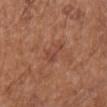{
  "biopsy_status": "not biopsied; imaged during a skin examination",
  "image": {
    "source": "total-body photography crop",
    "field_of_view_mm": 15
  },
  "lesion_size": {
    "long_diameter_mm_approx": 3.5
  },
  "patient": {
    "sex": "female",
    "age_approx": 75
  },
  "lighting": "white-light",
  "site": "chest",
  "automated_metrics": {
    "border_irregularity_0_10": 6.0,
    "color_variation_0_10": 2.0,
    "peripheral_color_asymmetry": 1.0,
    "nevus_likeness_0_100": 0,
    "lesion_detection_confidence_0_100": 100
  }
}